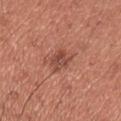Q: What are the patient's age and sex?
A: male, aged approximately 35
Q: Lesion size?
A: ~3.5 mm (longest diameter)
Q: What is the anatomic site?
A: the chest
Q: How was the tile lit?
A: white-light
Q: What kind of image is this?
A: ~15 mm tile from a whole-body skin photo
Q: Automated lesion metrics?
A: a lesion color around L≈48 a*≈26 b*≈28 in CIELAB and about 10 CIELAB-L* units darker than the surrounding skin; a border-irregularity index near 2.5/10, a color-variation rating of about 4/10, and radial color variation of about 1.5; a detector confidence of about 100 out of 100 that the crop contains a lesion